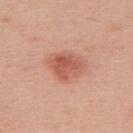A roughly 15 mm field-of-view crop from a total-body skin photograph. A female subject aged 63 to 67. The lesion is on the upper back.